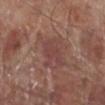{"biopsy_status": "not biopsied; imaged during a skin examination", "patient": {"sex": "male", "age_approx": 70}, "site": "leg", "lesion_size": {"long_diameter_mm_approx": 4.5}, "image": {"source": "total-body photography crop", "field_of_view_mm": 15}, "lighting": "white-light"}